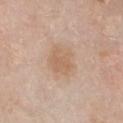Imaged with white-light lighting. Longest diameter approximately 3.5 mm. A 15 mm close-up extracted from a 3D total-body photography capture. The lesion is on the chest. Automated tile analysis of the lesion measured an average lesion color of about L≈61 a*≈17 b*≈32 (CIELAB), a lesion–skin lightness drop of about 7, and a normalized border contrast of about 5.5. The software also gave border irregularity of about 3 on a 0–10 scale, internal color variation of about 1.5 on a 0–10 scale, and radial color variation of about 0.5. A female subject in their mid-60s.